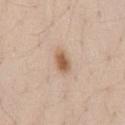- follow-up — no biopsy performed (imaged during a skin exam)
- lesion diameter — about 3 mm
- subject — male, about 35 years old
- imaging modality — total-body-photography crop, ~15 mm field of view
- lighting — white-light illumination
- site — the lower back